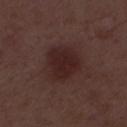Notes:
• subject: male, aged approximately 50
• acquisition: 15 mm crop, total-body photography